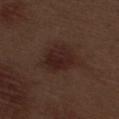biopsy_status: not biopsied; imaged during a skin examination
lighting: white-light
lesion_size:
  long_diameter_mm_approx: 6.0
site: right thigh
image:
  source: total-body photography crop
  field_of_view_mm: 15
patient:
  sex: male
  age_approx: 70